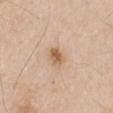Notes:
• notes: no biopsy performed (imaged during a skin exam)
• diameter: about 2.5 mm
• patient: male, aged around 65
• anatomic site: the right upper arm
• image: ~15 mm tile from a whole-body skin photo
• TBP lesion metrics: an average lesion color of about L≈61 a*≈18 b*≈35 (CIELAB), a lesion–skin lightness drop of about 11, and a normalized border contrast of about 8
• lighting: white-light illumination